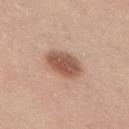Impression:
No biopsy was performed on this lesion — it was imaged during a full skin examination and was not determined to be concerning.
Acquisition and patient details:
Captured under white-light illumination. The lesion is on the back. Cropped from a whole-body photographic skin survey; the tile spans about 15 mm. About 4.5 mm across. A male patient aged approximately 75.